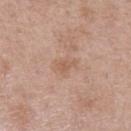This lesion was catalogued during total-body skin photography and was not selected for biopsy. A region of skin cropped from a whole-body photographic capture, roughly 15 mm wide. The lesion is located on the left forearm. Imaged with white-light lighting. The patient is a female aged 38 to 42.